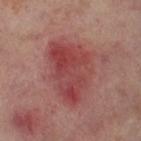biopsy_status: not biopsied; imaged during a skin examination
lesion_size:
  long_diameter_mm_approx: 7.5
site: leg
lighting: cross-polarized
patient:
  sex: female
  age_approx: 55
image:
  source: total-body photography crop
  field_of_view_mm: 15